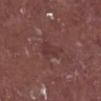Case summary:
• lesion diameter: ~3.5 mm (longest diameter)
• body site: the right lower leg
• automated lesion analysis: an average lesion color of about L≈35 a*≈22 b*≈20 (CIELAB), a lesion–skin lightness drop of about 5, and a normalized lesion–skin contrast near 5; a border-irregularity rating of about 4/10, a color-variation rating of about 1.5/10, and radial color variation of about 0.5; lesion-presence confidence of about 95/100
• patient: male, approximately 75 years of age
• image: ~15 mm crop, total-body skin-cancer survey
• tile lighting: white-light illumination